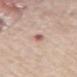notes — imaged on a skin check; not biopsied
subject — male, aged 73–77
location — the mid back
image-analysis metrics — an outline eccentricity of about 0.75 (0 = round, 1 = elongated) and two-axis asymmetry of about 0.15; a lesion–skin lightness drop of about 9 and a lesion-to-skin contrast of about 6 (normalized; higher = more distinct); border irregularity of about 2 on a 0–10 scale, internal color variation of about 7 on a 0–10 scale, and radial color variation of about 2
size — about 3 mm
image source — 15 mm crop, total-body photography
lighting — white-light illumination